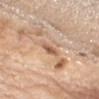Image and clinical context:
A close-up tile cropped from a whole-body skin photograph, about 15 mm across. Automated image analysis of the tile measured a lesion area of about 3 mm² and a shape eccentricity near 0.9. The analysis additionally found a mean CIELAB color near L≈59 a*≈20 b*≈33 and about 12 CIELAB-L* units darker than the surrounding skin. The analysis additionally found an automated nevus-likeness rating near 0 out of 100 and lesion-presence confidence of about 70/100. This is a white-light tile. Located on the right upper arm. A male subject aged 68 to 72.